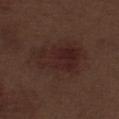Assessment:
The lesion was photographed on a routine skin check and not biopsied; there is no pathology result.
Background:
The tile uses white-light illumination. The lesion's longest dimension is about 7.5 mm. Cropped from a total-body skin-imaging series; the visible field is about 15 mm. A male subject aged approximately 70. The lesion is located on the abdomen.